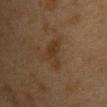Assessment: Part of a total-body skin-imaging series; this lesion was reviewed on a skin check and was not flagged for biopsy. Acquisition and patient details: Captured under cross-polarized illumination. On the chest. The patient is a female aged approximately 40. The total-body-photography lesion software estimated a lesion area of about 6.5 mm², a shape eccentricity near 0.8, and a shape-asymmetry score of about 0.55 (0 = symmetric). Measured at roughly 4 mm in maximum diameter. A 15 mm close-up extracted from a 3D total-body photography capture.